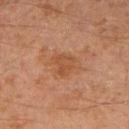Part of a total-body skin-imaging series; this lesion was reviewed on a skin check and was not flagged for biopsy.
A close-up tile cropped from a whole-body skin photograph, about 15 mm across.
Located on the right upper arm.
A male patient in their mid- to late 40s.
About 3 mm across.
The tile uses cross-polarized illumination.